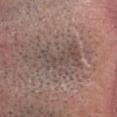biopsy status: imaged on a skin check; not biopsied
location: the head or neck
image: ~15 mm tile from a whole-body skin photo
lesion diameter: ~6.5 mm (longest diameter)
tile lighting: white-light
patient: male, aged 28 to 32